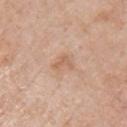Acquisition and patient details:
On the left upper arm. A male subject roughly 70 years of age. Imaged with white-light lighting. A region of skin cropped from a whole-body photographic capture, roughly 15 mm wide. Automated image analysis of the tile measured a footprint of about 3 mm² and two-axis asymmetry of about 0.6. It also reported a lesion color around L≈61 a*≈20 b*≈32 in CIELAB and a normalized border contrast of about 5.5. The analysis additionally found internal color variation of about 0 on a 0–10 scale.